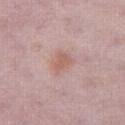The lesion was photographed on a routine skin check and not biopsied; there is no pathology result. On the right thigh. The subject is a female approximately 30 years of age. Cropped from a whole-body photographic skin survey; the tile spans about 15 mm. Captured under white-light illumination. The lesion's longest dimension is about 3 mm.